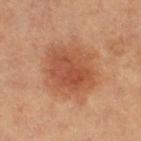follow-up = catalogued during a skin exam; not biopsied | TBP lesion metrics = a lesion area of about 26 mm²; a lesion color around L≈54 a*≈26 b*≈36 in CIELAB, a lesion–skin lightness drop of about 10, and a normalized border contrast of about 7 | patient = female, aged 58 to 62 | anatomic site = the right thigh | size = ≈6 mm | imaging modality = ~15 mm tile from a whole-body skin photo | illumination = cross-polarized illumination.